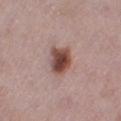Recorded during total-body skin imaging; not selected for excision or biopsy. This image is a 15 mm lesion crop taken from a total-body photograph. The lesion is located on the right thigh. A female patient, aged around 30.